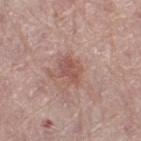This lesion was catalogued during total-body skin photography and was not selected for biopsy.
A close-up tile cropped from a whole-body skin photograph, about 15 mm across.
The lesion is located on the right thigh.
The lesion-visualizer software estimated an area of roughly 6 mm², an outline eccentricity of about 0.7 (0 = round, 1 = elongated), and a shape-asymmetry score of about 0.3 (0 = symmetric). And it measured a border-irregularity index near 3.5/10, a within-lesion color-variation index near 3/10, and a peripheral color-asymmetry measure near 1. The analysis additionally found an automated nevus-likeness rating near 60 out of 100 and a detector confidence of about 100 out of 100 that the crop contains a lesion.
The recorded lesion diameter is about 3.5 mm.
A female patient aged approximately 65.
This is a white-light tile.